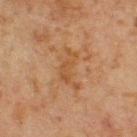Impression:
Recorded during total-body skin imaging; not selected for excision or biopsy.
Context:
The recorded lesion diameter is about 5 mm. A lesion tile, about 15 mm wide, cut from a 3D total-body photograph. Captured under cross-polarized illumination. A male patient, roughly 65 years of age. From the chest. An algorithmic analysis of the crop reported an eccentricity of roughly 0.85. It also reported a normalized border contrast of about 5.5.